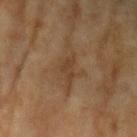Recorded during total-body skin imaging; not selected for excision or biopsy. A lesion tile, about 15 mm wide, cut from a 3D total-body photograph. The recorded lesion diameter is about 3 mm. The patient is a female aged approximately 60. Automated image analysis of the tile measured roughly 6 lightness units darker than nearby skin and a lesion-to-skin contrast of about 6 (normalized; higher = more distinct). The analysis additionally found a nevus-likeness score of about 0/100 and lesion-presence confidence of about 90/100. From the left upper arm. The tile uses cross-polarized illumination.